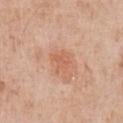The lesion was tiled from a total-body skin photograph and was not biopsied.